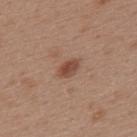This lesion was catalogued during total-body skin photography and was not selected for biopsy. This is a white-light tile. The subject is a female approximately 40 years of age. Located on the upper back. Cropped from a whole-body photographic skin survey; the tile spans about 15 mm.